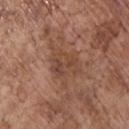This lesion was catalogued during total-body skin photography and was not selected for biopsy.
The lesion's longest dimension is about 5.5 mm.
A male patient aged approximately 70.
Captured under white-light illumination.
Automated image analysis of the tile measured a footprint of about 12 mm², an outline eccentricity of about 0.6 (0 = round, 1 = elongated), and a shape-asymmetry score of about 0.45 (0 = symmetric).
A region of skin cropped from a whole-body photographic capture, roughly 15 mm wide.
From the chest.